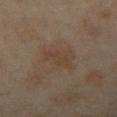This lesion was catalogued during total-body skin photography and was not selected for biopsy. The subject is a female aged 33 to 37. A roughly 15 mm field-of-view crop from a total-body skin photograph. From the left forearm. Captured under cross-polarized illumination. Approximately 3.5 mm at its widest.